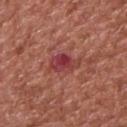workup=no biopsy performed (imaged during a skin exam)
subject=male, about 65 years old
lesion size=≈3.5 mm
anatomic site=the upper back
image=~15 mm crop, total-body skin-cancer survey